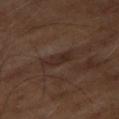Imaged during a routine full-body skin examination; the lesion was not biopsied and no histopathology is available. A male patient aged approximately 65. A 15 mm crop from a total-body photograph taken for skin-cancer surveillance. Approximately 4 mm at its widest. Captured under cross-polarized illumination. The lesion-visualizer software estimated a mean CIELAB color near L≈25 a*≈15 b*≈21, roughly 6 lightness units darker than nearby skin, and a normalized border contrast of about 6.5. And it measured border irregularity of about 4 on a 0–10 scale, internal color variation of about 1.5 on a 0–10 scale, and peripheral color asymmetry of about 0.5. It also reported a classifier nevus-likeness of about 0/100 and lesion-presence confidence of about 60/100.